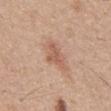Findings:
• biopsy status — no biopsy performed (imaged during a skin exam)
• tile lighting — white-light illumination
• acquisition — 15 mm crop, total-body photography
• subject — male, approximately 30 years of age
• body site — the front of the torso